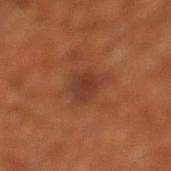Q: Is there a histopathology result?
A: total-body-photography surveillance lesion; no biopsy
Q: Where on the body is the lesion?
A: the left lower leg
Q: How was this image acquired?
A: ~15 mm tile from a whole-body skin photo
Q: Patient demographics?
A: roughly 65 years of age